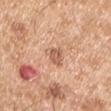| key | value |
|---|---|
| workup | imaged on a skin check; not biopsied |
| acquisition | ~15 mm tile from a whole-body skin photo |
| tile lighting | white-light |
| TBP lesion metrics | a border-irregularity rating of about 3.5/10 and a color-variation rating of about 2/10 |
| patient | male, aged 53–57 |
| location | the left upper arm |